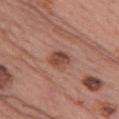{
  "biopsy_status": "not biopsied; imaged during a skin examination",
  "site": "front of the torso",
  "image": {
    "source": "total-body photography crop",
    "field_of_view_mm": 15
  },
  "patient": {
    "sex": "female",
    "age_approx": 60
  }
}